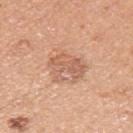biopsy_status: not biopsied; imaged during a skin examination
site: left upper arm
patient:
  sex: male
  age_approx: 45
lesion_size:
  long_diameter_mm_approx: 4.0
lighting: white-light
image:
  source: total-body photography crop
  field_of_view_mm: 15